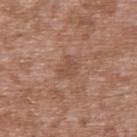follow-up — no biopsy performed (imaged during a skin exam) | imaging modality — ~15 mm tile from a whole-body skin photo | tile lighting — white-light illumination | subject — male, aged 43 to 47 | site — the upper back | automated metrics — a footprint of about 4 mm², an outline eccentricity of about 0.55 (0 = round, 1 = elongated), and a symmetry-axis asymmetry near 0.4; a border-irregularity rating of about 4/10 and radial color variation of about 0.5; a nevus-likeness score of about 0/100 and lesion-presence confidence of about 100/100 | size — ~2.5 mm (longest diameter).